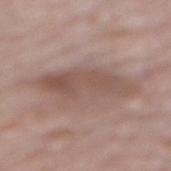Q: How was this image acquired?
A: 15 mm crop, total-body photography
Q: Where on the body is the lesion?
A: the mid back
Q: Patient demographics?
A: male, approximately 85 years of age or older
Q: What lighting was used for the tile?
A: white-light illumination